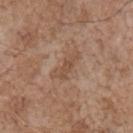Part of a total-body skin-imaging series; this lesion was reviewed on a skin check and was not flagged for biopsy. About 4 mm across. Located on the right upper arm. A 15 mm close-up extracted from a 3D total-body photography capture. A male subject, roughly 70 years of age.